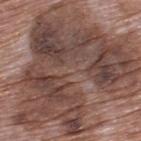illumination: white-light | size: ~15.5 mm (longest diameter) | image: total-body-photography crop, ~15 mm field of view | location: the upper back | patient: male, about 70 years old.